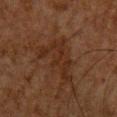{
  "automated_metrics": {
    "area_mm2_approx": 15.0,
    "eccentricity": 0.7,
    "shape_asymmetry": 0.6,
    "nevus_likeness_0_100": 0,
    "lesion_detection_confidence_0_100": 90
  },
  "site": "chest",
  "patient": {
    "sex": "male",
    "age_approx": 65
  },
  "image": {
    "source": "total-body photography crop",
    "field_of_view_mm": 15
  },
  "lesion_size": {
    "long_diameter_mm_approx": 6.0
  },
  "lighting": "cross-polarized"
}